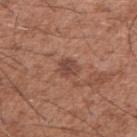Part of a total-body skin-imaging series; this lesion was reviewed on a skin check and was not flagged for biopsy.
Automated tile analysis of the lesion measured a border-irregularity rating of about 2.5/10, a within-lesion color-variation index near 3/10, and peripheral color asymmetry of about 1. The analysis additionally found a nevus-likeness score of about 50/100.
The lesion is on the right upper arm.
Captured under white-light illumination.
A male subject, roughly 50 years of age.
A 15 mm crop from a total-body photograph taken for skin-cancer surveillance.
The lesion's longest dimension is about 2.5 mm.